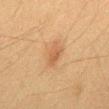Clinical impression: The lesion was tiled from a total-body skin photograph and was not biopsied. Image and clinical context: The lesion's longest dimension is about 3 mm. A lesion tile, about 15 mm wide, cut from a 3D total-body photograph. Captured under cross-polarized illumination. Automated image analysis of the tile measured a shape eccentricity near 0.8. The analysis additionally found an average lesion color of about L≈45 a*≈17 b*≈30 (CIELAB) and roughly 7 lightness units darker than nearby skin. On the mid back. A male subject, aged 33 to 37.